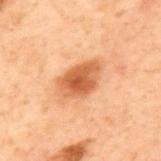Impression:
Recorded during total-body skin imaging; not selected for excision or biopsy.
Clinical summary:
A close-up tile cropped from a whole-body skin photograph, about 15 mm across. A male subject in their 70s. Located on the back. Measured at roughly 5 mm in maximum diameter. An algorithmic analysis of the crop reported an area of roughly 11 mm² and an eccentricity of roughly 0.8. The analysis additionally found a lesion color around L≈46 a*≈23 b*≈33 in CIELAB, about 11 CIELAB-L* units darker than the surrounding skin, and a normalized border contrast of about 9. It also reported a classifier nevus-likeness of about 95/100. Captured under cross-polarized illumination.